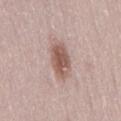Findings:
– patient: female, aged around 50
– TBP lesion metrics: an average lesion color of about L≈55 a*≈19 b*≈24 (CIELAB) and a normalized border contrast of about 9
– lighting: white-light
– imaging modality: 15 mm crop, total-body photography
– lesion size: about 4 mm
– site: the lower back